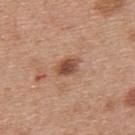{
  "biopsy_status": "not biopsied; imaged during a skin examination",
  "patient": {
    "sex": "male",
    "age_approx": 30
  },
  "automated_metrics": {
    "area_mm2_approx": 4.5,
    "shape_asymmetry": 0.15
  },
  "image": {
    "source": "total-body photography crop",
    "field_of_view_mm": 15
  },
  "lesion_size": {
    "long_diameter_mm_approx": 3.0
  },
  "lighting": "white-light",
  "site": "back"
}